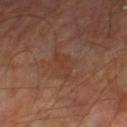Recorded during total-body skin imaging; not selected for excision or biopsy. A 15 mm crop from a total-body photograph taken for skin-cancer surveillance. This is a cross-polarized tile. On the right lower leg. The lesion's longest dimension is about 4 mm. An algorithmic analysis of the crop reported a lesion area of about 4.5 mm², an outline eccentricity of about 0.9 (0 = round, 1 = elongated), and two-axis asymmetry of about 0.4. The software also gave roughly 5 lightness units darker than nearby skin and a normalized border contrast of about 5. And it measured a classifier nevus-likeness of about 0/100 and lesion-presence confidence of about 100/100. A male patient, aged around 70.